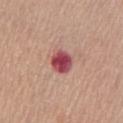Clinical impression:
The lesion was tiled from a total-body skin photograph and was not biopsied.
Acquisition and patient details:
A female subject, aged approximately 65. The lesion-visualizer software estimated an area of roughly 7.5 mm², an eccentricity of roughly 0.65, and a shape-asymmetry score of about 0.2 (0 = symmetric). And it measured a lesion–skin lightness drop of about 16 and a normalized border contrast of about 11.5. The lesion is on the chest. The lesion's longest dimension is about 3.5 mm. The tile uses white-light illumination. A 15 mm crop from a total-body photograph taken for skin-cancer surveillance.